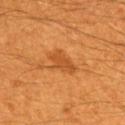Findings:
– diameter · ≈3.5 mm
– lighting · cross-polarized
– body site · the upper back
– automated lesion analysis · a lesion–skin lightness drop of about 9 and a normalized lesion–skin contrast near 6.5
– patient · male, aged approximately 60
– imaging modality · ~15 mm tile from a whole-body skin photo